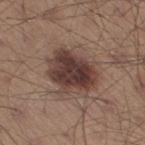biopsy_status: not biopsied; imaged during a skin examination
patient:
  sex: male
  age_approx: 30
site: right thigh
image:
  source: total-body photography crop
  field_of_view_mm: 15